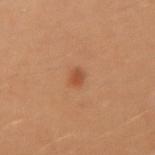{
  "biopsy_status": "not biopsied; imaged during a skin examination",
  "site": "right upper arm",
  "image": {
    "source": "total-body photography crop",
    "field_of_view_mm": 15
  },
  "automated_metrics": {
    "eccentricity": 0.7,
    "shape_asymmetry": 0.15,
    "border_irregularity_0_10": 1.5,
    "color_variation_0_10": 1.5,
    "peripheral_color_asymmetry": 0.5,
    "nevus_likeness_0_100": 90,
    "lesion_detection_confidence_0_100": 100
  },
  "lesion_size": {
    "long_diameter_mm_approx": 2.0
  },
  "patient": {
    "sex": "male",
    "age_approx": 30
  }
}